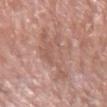notes: imaged on a skin check; not biopsied
subject: male, aged approximately 80
automated lesion analysis: a classifier nevus-likeness of about 0/100 and lesion-presence confidence of about 90/100
image source: ~15 mm tile from a whole-body skin photo
size: ~7.5 mm (longest diameter)
tile lighting: white-light illumination
site: the left forearm